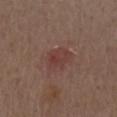notes: catalogued during a skin exam; not biopsied | image source: ~15 mm crop, total-body skin-cancer survey | subject: male, aged around 70 | illumination: white-light illumination | TBP lesion metrics: an average lesion color of about L≈39 a*≈21 b*≈22 (CIELAB) and roughly 6 lightness units darker than nearby skin; border irregularity of about 2 on a 0–10 scale and a within-lesion color-variation index near 3.5/10 | site: the right upper arm | size: ~3.5 mm (longest diameter).